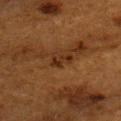No biopsy was performed on this lesion — it was imaged during a full skin examination and was not determined to be concerning. The subject is a female approximately 50 years of age. A roughly 15 mm field-of-view crop from a total-body skin photograph. From the left upper arm. The total-body-photography lesion software estimated a mean CIELAB color near L≈23 a*≈19 b*≈27, a lesion–skin lightness drop of about 7, and a lesion-to-skin contrast of about 8.5 (normalized; higher = more distinct). The software also gave a classifier nevus-likeness of about 0/100 and a detector confidence of about 95 out of 100 that the crop contains a lesion.